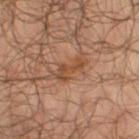– anatomic site — the right thigh
– patient — male, aged approximately 65
– acquisition — ~15 mm tile from a whole-body skin photo